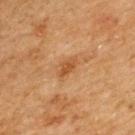notes — catalogued during a skin exam; not biopsied
image source — 15 mm crop, total-body photography
image-analysis metrics — a mean CIELAB color near L≈41 a*≈20 b*≈34, a lesion–skin lightness drop of about 8, and a normalized border contrast of about 7
tile lighting — cross-polarized
patient — male, aged around 50
size — about 2.5 mm
site — the back